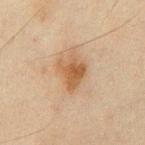illumination = cross-polarized illumination | TBP lesion metrics = a classifier nevus-likeness of about 45/100 and a detector confidence of about 100 out of 100 that the crop contains a lesion | diameter = about 3.5 mm | imaging modality = ~15 mm tile from a whole-body skin photo | anatomic site = the abdomen | subject = male, approximately 45 years of age.